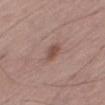This image is a 15 mm lesion crop taken from a total-body photograph. A male subject aged 63 to 67. The lesion is on the leg.